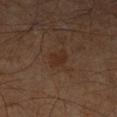Captured during whole-body skin photography for melanoma surveillance; the lesion was not biopsied. An algorithmic analysis of the crop reported an eccentricity of roughly 0.75. Captured under cross-polarized illumination. About 2.5 mm across. A 15 mm crop from a total-body photograph taken for skin-cancer surveillance. A male patient about 60 years old. The lesion is on the leg.